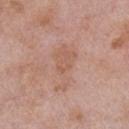This lesion was catalogued during total-body skin photography and was not selected for biopsy.
Located on the right upper arm.
This is a white-light tile.
Longest diameter approximately 5.5 mm.
Automated image analysis of the tile measured a border-irregularity rating of about 6.5/10, a within-lesion color-variation index near 2/10, and radial color variation of about 1. The analysis additionally found a nevus-likeness score of about 0/100 and lesion-presence confidence of about 100/100.
A male subject roughly 55 years of age.
A region of skin cropped from a whole-body photographic capture, roughly 15 mm wide.